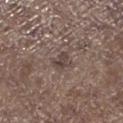{"lesion_size": {"long_diameter_mm_approx": 2.5}, "image": {"source": "total-body photography crop", "field_of_view_mm": 15}, "site": "left lower leg", "patient": {"sex": "male", "age_approx": 70}, "automated_metrics": {"area_mm2_approx": 3.5, "eccentricity": 0.75, "shape_asymmetry": 0.35, "nevus_likeness_0_100": 0, "lesion_detection_confidence_0_100": 75}}